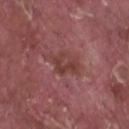follow-up: catalogued during a skin exam; not biopsied | lighting: white-light | acquisition: ~15 mm tile from a whole-body skin photo | body site: the left forearm | subject: male, about 40 years old.